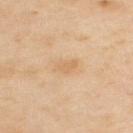Clinical impression:
The lesion was photographed on a routine skin check and not biopsied; there is no pathology result.
Context:
A 15 mm close-up extracted from a 3D total-body photography capture. A male subject, approximately 55 years of age. On the upper back. This is a cross-polarized tile. An algorithmic analysis of the crop reported a within-lesion color-variation index near 1.5/10 and peripheral color asymmetry of about 0.5.